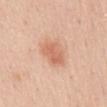Imaged during a routine full-body skin examination; the lesion was not biopsied and no histopathology is available.
A female patient, aged approximately 45.
On the mid back.
Captured under white-light illumination.
A region of skin cropped from a whole-body photographic capture, roughly 15 mm wide.
About 3.5 mm across.